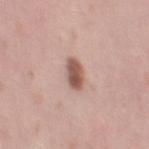{
  "biopsy_status": "not biopsied; imaged during a skin examination",
  "site": "leg",
  "patient": {
    "sex": "female",
    "age_approx": 40
  },
  "automated_metrics": {
    "area_mm2_approx": 5.0,
    "eccentricity": 0.85,
    "cielab_L": 55,
    "cielab_a": 21,
    "cielab_b": 25,
    "vs_skin_darker_L": 15.0,
    "vs_skin_contrast_norm": 9.5,
    "border_irregularity_0_10": 2.5,
    "color_variation_0_10": 3.5,
    "peripheral_color_asymmetry": 1.5
  },
  "lighting": "white-light",
  "image": {
    "source": "total-body photography crop",
    "field_of_view_mm": 15
  }
}